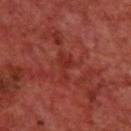No biopsy was performed on this lesion — it was imaged during a full skin examination and was not determined to be concerning. Located on the upper back. A male subject approximately 70 years of age. Cropped from a whole-body photographic skin survey; the tile spans about 15 mm.